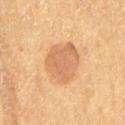Q: Is there a histopathology result?
A: total-body-photography surveillance lesion; no biopsy
Q: What is the lesion's diameter?
A: about 5 mm
Q: How was the tile lit?
A: cross-polarized
Q: What kind of image is this?
A: ~15 mm tile from a whole-body skin photo
Q: Where on the body is the lesion?
A: the lower back
Q: Automated lesion metrics?
A: an average lesion color of about L≈56 a*≈19 b*≈34 (CIELAB) and a lesion-to-skin contrast of about 6 (normalized; higher = more distinct)
Q: Patient demographics?
A: male, in their mid-80s or older